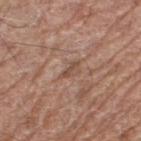Case summary:
• workup — catalogued during a skin exam; not biopsied
• body site — the leg
• automated metrics — an average lesion color of about L≈49 a*≈19 b*≈27 (CIELAB) and a normalized border contrast of about 6; an automated nevus-likeness rating near 0 out of 100 and lesion-presence confidence of about 75/100
• illumination — white-light illumination
• acquisition — ~15 mm tile from a whole-body skin photo
• patient — male, aged 68 to 72
• lesion diameter — ≈2.5 mm